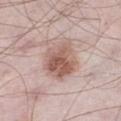Impression:
No biopsy was performed on this lesion — it was imaged during a full skin examination and was not determined to be concerning.
Context:
The lesion is on the left thigh. Longest diameter approximately 5 mm. Automated tile analysis of the lesion measured an outline eccentricity of about 0.65 (0 = round, 1 = elongated) and two-axis asymmetry of about 0.2. The software also gave a lesion–skin lightness drop of about 12 and a normalized border contrast of about 8.5. The analysis additionally found a nevus-likeness score of about 85/100 and a detector confidence of about 100 out of 100 that the crop contains a lesion. The patient is a male aged around 55. A lesion tile, about 15 mm wide, cut from a 3D total-body photograph. Imaged with white-light lighting.